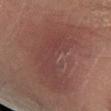{
  "biopsy_status": "not biopsied; imaged during a skin examination",
  "image": {
    "source": "total-body photography crop",
    "field_of_view_mm": 15
  },
  "site": "left lower leg",
  "patient": {
    "sex": "female",
    "age_approx": 50
  }
}